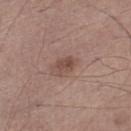biopsy status: catalogued during a skin exam; not biopsied
tile lighting: white-light
patient: male, in their 70s
body site: the left lower leg
imaging modality: ~15 mm crop, total-body skin-cancer survey
lesion diameter: ~2.5 mm (longest diameter)
automated metrics: a footprint of about 4 mm², an eccentricity of roughly 0.65, and a shape-asymmetry score of about 0.3 (0 = symmetric); an average lesion color of about L≈48 a*≈19 b*≈24 (CIELAB), a lesion–skin lightness drop of about 9, and a normalized lesion–skin contrast near 7; an automated nevus-likeness rating near 40 out of 100 and a detector confidence of about 100 out of 100 that the crop contains a lesion